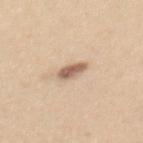Findings:
- tile lighting — white-light illumination
- size — ~3 mm (longest diameter)
- patient — female, aged 23–27
- site — the mid back
- image — ~15 mm tile from a whole-body skin photo
- automated metrics — a lesion color around L≈61 a*≈18 b*≈29 in CIELAB, about 15 CIELAB-L* units darker than the surrounding skin, and a normalized lesion–skin contrast near 9